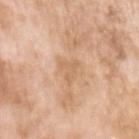lighting: white-light illumination
body site: the left upper arm
image-analysis metrics: a footprint of about 4 mm², an eccentricity of roughly 0.6, and a shape-asymmetry score of about 0.5 (0 = symmetric); a lesion color around L≈64 a*≈19 b*≈35 in CIELAB and roughly 7 lightness units darker than nearby skin; a border-irregularity index near 5.5/10 and a peripheral color-asymmetry measure near 0.5; an automated nevus-likeness rating near 0 out of 100 and a detector confidence of about 100 out of 100 that the crop contains a lesion
acquisition: ~15 mm crop, total-body skin-cancer survey
patient: female, roughly 75 years of age
lesion size: about 3 mm